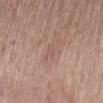No biopsy was performed on this lesion — it was imaged during a full skin examination and was not determined to be concerning.
Cropped from a total-body skin-imaging series; the visible field is about 15 mm.
About 2 mm across.
The patient is a female aged 63 to 67.
Imaged with white-light lighting.
The lesion is on the mid back.